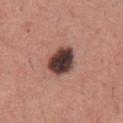No biopsy was performed on this lesion — it was imaged during a full skin examination and was not determined to be concerning. A male patient aged 43–47. Approximately 4 mm at its widest. Located on the chest. Cropped from a total-body skin-imaging series; the visible field is about 15 mm. This is a white-light tile.